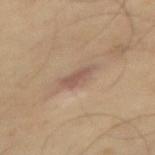{
  "biopsy_status": "not biopsied; imaged during a skin examination",
  "patient": {
    "sex": "male",
    "age_approx": 65
  },
  "image": {
    "source": "total-body photography crop",
    "field_of_view_mm": 15
  }
}